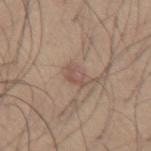Part of a total-body skin-imaging series; this lesion was reviewed on a skin check and was not flagged for biopsy.
Cropped from a total-body skin-imaging series; the visible field is about 15 mm.
A male patient aged approximately 65.
Located on the abdomen.
Imaged with white-light lighting.
Measured at roughly 3 mm in maximum diameter.